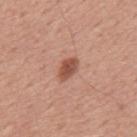  biopsy_status: not biopsied; imaged during a skin examination
  patient:
    sex: male
    age_approx: 50
  image:
    source: total-body photography crop
    field_of_view_mm: 15
  lighting: white-light
  automated_metrics:
    area_mm2_approx: 4.5
    eccentricity: 0.8
    shape_asymmetry: 0.2
    border_irregularity_0_10: 2.0
    peripheral_color_asymmetry: 0.5
  site: mid back